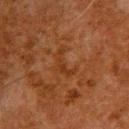A 15 mm crop from a total-body photograph taken for skin-cancer surveillance. Approximately 3.5 mm at its widest. Captured under cross-polarized illumination. Located on the upper back. Automated image analysis of the tile measured an eccentricity of roughly 0.9 and two-axis asymmetry of about 0.75. The software also gave a mean CIELAB color near L≈27 a*≈20 b*≈30 and a normalized border contrast of about 5.5. It also reported a border-irregularity rating of about 8.5/10 and a color-variation rating of about 0/10. A male subject, about 60 years old.